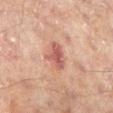biopsy_status: not biopsied; imaged during a skin examination
lighting: cross-polarized
image:
  source: total-body photography crop
  field_of_view_mm: 15
patient:
  sex: male
  age_approx: 65
lesion_size:
  long_diameter_mm_approx: 3.0
automated_metrics:
  area_mm2_approx: 6.0
  eccentricity: 0.7
  border_irregularity_0_10: 3.0
  color_variation_0_10: 2.5
  peripheral_color_asymmetry: 1.0
  nevus_likeness_0_100: 0
  lesion_detection_confidence_0_100: 100
site: leg